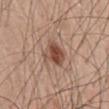Clinical impression:
The lesion was photographed on a routine skin check and not biopsied; there is no pathology result.
Background:
Cropped from a total-body skin-imaging series; the visible field is about 15 mm. A male subject approximately 50 years of age. The lesion is on the mid back. Imaged with white-light lighting. The recorded lesion diameter is about 3.5 mm.